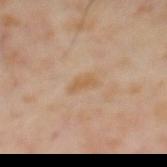Notes:
* follow-up — no biopsy performed (imaged during a skin exam)
* lesion diameter — ~2.5 mm (longest diameter)
* lighting — cross-polarized
* automated lesion analysis — a lesion area of about 3 mm² and a shape-asymmetry score of about 0.35 (0 = symmetric); border irregularity of about 3.5 on a 0–10 scale and a color-variation rating of about 1/10; a classifier nevus-likeness of about 0/100 and lesion-presence confidence of about 100/100
* subject — male, in their mid- to late 60s
* image — 15 mm crop, total-body photography